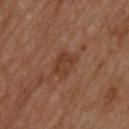Part of a total-body skin-imaging series; this lesion was reviewed on a skin check and was not flagged for biopsy. Imaged with cross-polarized lighting. A close-up tile cropped from a whole-body skin photograph, about 15 mm across. From the upper back. Approximately 3 mm at its widest. A male patient, approximately 65 years of age.